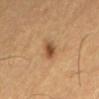The lesion was photographed on a routine skin check and not biopsied; there is no pathology result. Automated image analysis of the tile measured a lesion area of about 4 mm², a shape eccentricity near 0.8, and a symmetry-axis asymmetry near 0.25. It also reported a border-irregularity rating of about 2.5/10, a color-variation rating of about 2.5/10, and radial color variation of about 1. And it measured lesion-presence confidence of about 100/100. From the lower back. Approximately 3 mm at its widest. A male patient aged 58–62. Captured under cross-polarized illumination. A close-up tile cropped from a whole-body skin photograph, about 15 mm across.